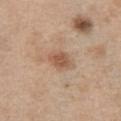No biopsy was performed on this lesion — it was imaged during a full skin examination and was not determined to be concerning.
Approximately 3 mm at its widest.
The lesion is located on the chest.
A female patient, aged around 40.
Captured under white-light illumination.
A close-up tile cropped from a whole-body skin photograph, about 15 mm across.
An algorithmic analysis of the crop reported a lesion area of about 5.5 mm², an outline eccentricity of about 0.65 (0 = round, 1 = elongated), and a shape-asymmetry score of about 0.2 (0 = symmetric). It also reported a lesion color around L≈55 a*≈20 b*≈31 in CIELAB, roughly 10 lightness units darker than nearby skin, and a normalized border contrast of about 7.